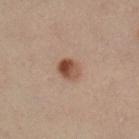No biopsy was performed on this lesion — it was imaged during a full skin examination and was not determined to be concerning. The lesion is on the right lower leg. The subject is a female in their 40s. Captured under cross-polarized illumination. A close-up tile cropped from a whole-body skin photograph, about 15 mm across.